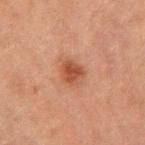biopsy status: catalogued during a skin exam; not biopsied | image-analysis metrics: a mean CIELAB color near L≈40 a*≈23 b*≈29, a lesion–skin lightness drop of about 9, and a normalized lesion–skin contrast near 8.5; an automated nevus-likeness rating near 95 out of 100 and a detector confidence of about 100 out of 100 that the crop contains a lesion | site: the mid back | patient: male, about 75 years old | imaging modality: 15 mm crop, total-body photography | tile lighting: cross-polarized illumination.